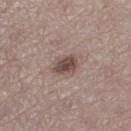{"biopsy_status": "not biopsied; imaged during a skin examination", "patient": {"sex": "female", "age_approx": 50}, "image": {"source": "total-body photography crop", "field_of_view_mm": 15}, "site": "leg"}